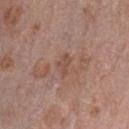Findings:
- follow-up · total-body-photography surveillance lesion; no biopsy
- patient · male, approximately 70 years of age
- illumination · white-light
- TBP lesion metrics · an area of roughly 3 mm² and an outline eccentricity of about 0.8 (0 = round, 1 = elongated)
- anatomic site · the chest
- acquisition · ~15 mm tile from a whole-body skin photo
- lesion diameter · about 2.5 mm